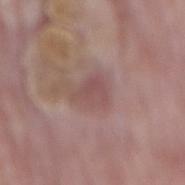Part of a total-body skin-imaging series; this lesion was reviewed on a skin check and was not flagged for biopsy. Captured under white-light illumination. A roughly 15 mm field-of-view crop from a total-body skin photograph. The lesion is located on the mid back. Automated tile analysis of the lesion measured an average lesion color of about L≈50 a*≈20 b*≈20 (CIELAB), about 7 CIELAB-L* units darker than the surrounding skin, and a normalized lesion–skin contrast near 5. The analysis additionally found a lesion-detection confidence of about 100/100. Approximately 3.5 mm at its widest. The patient is a male roughly 85 years of age or older.